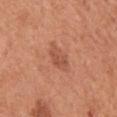The lesion was tiled from a total-body skin photograph and was not biopsied. From the chest. Imaged with white-light lighting. An algorithmic analysis of the crop reported a shape eccentricity near 0.8 and a symmetry-axis asymmetry near 0.25. The analysis additionally found a mean CIELAB color near L≈53 a*≈26 b*≈33, about 8 CIELAB-L* units darker than the surrounding skin, and a normalized lesion–skin contrast near 5.5. A 15 mm close-up extracted from a 3D total-body photography capture. A male subject, approximately 65 years of age. The lesion's longest dimension is about 3.5 mm.